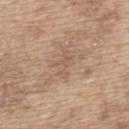Findings:
- workup — total-body-photography surveillance lesion; no biopsy
- body site — the upper back
- image source — total-body-photography crop, ~15 mm field of view
- lighting — white-light illumination
- subject — male, aged 63 to 67